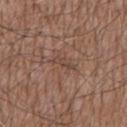This lesion was catalogued during total-body skin photography and was not selected for biopsy.
The total-body-photography lesion software estimated a lesion color around L≈44 a*≈17 b*≈24 in CIELAB and about 7 CIELAB-L* units darker than the surrounding skin.
The subject is a male about 75 years old.
A lesion tile, about 15 mm wide, cut from a 3D total-body photograph.
Measured at roughly 3 mm in maximum diameter.
Located on the back.
Imaged with white-light lighting.